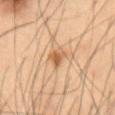Part of a total-body skin-imaging series; this lesion was reviewed on a skin check and was not flagged for biopsy. A male patient aged 53 to 57. Located on the abdomen. A region of skin cropped from a whole-body photographic capture, roughly 15 mm wide.